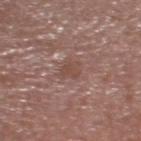Imaged during a routine full-body skin examination; the lesion was not biopsied and no histopathology is available. The lesion is on the head or neck. Measured at roughly 2.5 mm in maximum diameter. A close-up tile cropped from a whole-body skin photograph, about 15 mm across. A male patient roughly 65 years of age.